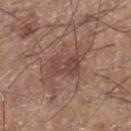Imaged during a routine full-body skin examination; the lesion was not biopsied and no histopathology is available.
The lesion is located on the right lower leg.
A lesion tile, about 15 mm wide, cut from a 3D total-body photograph.
Imaged with white-light lighting.
The patient is a male aged 58 to 62.
An algorithmic analysis of the crop reported roughly 8 lightness units darker than nearby skin and a lesion-to-skin contrast of about 6.5 (normalized; higher = more distinct). The analysis additionally found a classifier nevus-likeness of about 0/100 and a lesion-detection confidence of about 100/100.